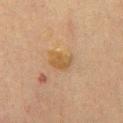* follow-up · imaged on a skin check; not biopsied
* tile lighting · cross-polarized
* TBP lesion metrics · a lesion area of about 5.5 mm², an eccentricity of roughly 0.6, and two-axis asymmetry of about 0.25; an average lesion color of about L≈44 a*≈14 b*≈32 (CIELAB) and about 6 CIELAB-L* units darker than the surrounding skin; an automated nevus-likeness rating near 0 out of 100 and lesion-presence confidence of about 100/100
* patient · male, aged around 65
* body site · the chest
* acquisition · total-body-photography crop, ~15 mm field of view
* lesion diameter · ~3 mm (longest diameter)